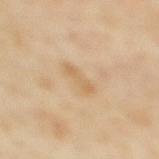Recorded during total-body skin imaging; not selected for excision or biopsy.
The patient is a female about 35 years old.
The lesion's longest dimension is about 3.5 mm.
On the upper back.
Imaged with cross-polarized lighting.
A region of skin cropped from a whole-body photographic capture, roughly 15 mm wide.